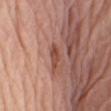| key | value |
|---|---|
| follow-up | catalogued during a skin exam; not biopsied |
| patient | male, roughly 80 years of age |
| imaging modality | 15 mm crop, total-body photography |
| site | the right upper arm |